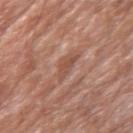No biopsy was performed on this lesion — it was imaged during a full skin examination and was not determined to be concerning.
The lesion is located on the left upper arm.
Captured under white-light illumination.
A 15 mm crop from a total-body photograph taken for skin-cancer surveillance.
Measured at roughly 3 mm in maximum diameter.
The patient is a male in their 80s.